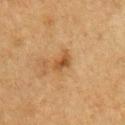Impression:
Part of a total-body skin-imaging series; this lesion was reviewed on a skin check and was not flagged for biopsy.
Acquisition and patient details:
Approximately 2.5 mm at its widest. The lesion is located on the arm. This image is a 15 mm lesion crop taken from a total-body photograph. A male patient aged 73–77.